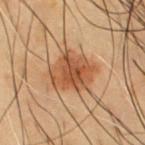About 4.5 mm across. This image is a 15 mm lesion crop taken from a total-body photograph. Captured under cross-polarized illumination. A male subject, roughly 55 years of age. Automated image analysis of the tile measured a lesion–skin lightness drop of about 10 and a lesion-to-skin contrast of about 8.5 (normalized; higher = more distinct). The analysis additionally found border irregularity of about 3 on a 0–10 scale, internal color variation of about 4.5 on a 0–10 scale, and radial color variation of about 1.5. It also reported an automated nevus-likeness rating near 85 out of 100. The lesion is on the chest.